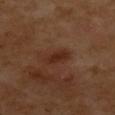Imaged during a routine full-body skin examination; the lesion was not biopsied and no histopathology is available.
This is a cross-polarized tile.
Longest diameter approximately 3 mm.
From the upper back.
A 15 mm crop from a total-body photograph taken for skin-cancer surveillance.
An algorithmic analysis of the crop reported a mean CIELAB color near L≈29 a*≈22 b*≈28, roughly 7 lightness units darker than nearby skin, and a normalized border contrast of about 8. It also reported border irregularity of about 2 on a 0–10 scale. The software also gave lesion-presence confidence of about 100/100.
A female patient, about 55 years old.